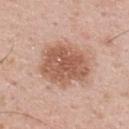Q: Was this lesion biopsied?
A: imaged on a skin check; not biopsied
Q: What did automated image analysis measure?
A: a mean CIELAB color near L≈58 a*≈22 b*≈29, about 12 CIELAB-L* units darker than the surrounding skin, and a normalized lesion–skin contrast near 8; border irregularity of about 2 on a 0–10 scale, a color-variation rating of about 3.5/10, and radial color variation of about 1.5; an automated nevus-likeness rating near 15 out of 100 and a detector confidence of about 100 out of 100 that the crop contains a lesion
Q: Patient demographics?
A: male, about 55 years old
Q: Lesion location?
A: the back
Q: What is the lesion's diameter?
A: ~6 mm (longest diameter)
Q: What is the imaging modality?
A: 15 mm crop, total-body photography